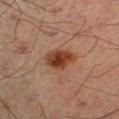<case>
<biopsy_status>not biopsied; imaged during a skin examination</biopsy_status>
<image>
  <source>total-body photography crop</source>
  <field_of_view_mm>15</field_of_view_mm>
</image>
<site>left leg</site>
<patient>
  <sex>male</sex>
  <age_approx>50</age_approx>
</patient>
<lighting>cross-polarized</lighting>
</case>